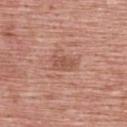{"biopsy_status": "not biopsied; imaged during a skin examination"}